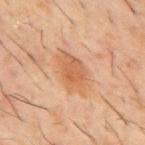{"biopsy_status": "not biopsied; imaged during a skin examination", "lesion_size": {"long_diameter_mm_approx": 5.5}, "lighting": "cross-polarized", "site": "back", "image": {"source": "total-body photography crop", "field_of_view_mm": 15}, "patient": {"sex": "male", "age_approx": 60}, "automated_metrics": {"cielab_L": 57, "cielab_a": 23, "cielab_b": 35, "vs_skin_darker_L": 8.0, "vs_skin_contrast_norm": 6.5, "peripheral_color_asymmetry": 1.0, "lesion_detection_confidence_0_100": 100}}